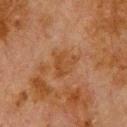workup: catalogued during a skin exam; not biopsied
size: ~3.5 mm (longest diameter)
image source: ~15 mm crop, total-body skin-cancer survey
automated metrics: about 6 CIELAB-L* units darker than the surrounding skin; a border-irregularity rating of about 5.5/10, a within-lesion color-variation index near 2/10, and radial color variation of about 0.5
tile lighting: cross-polarized illumination
subject: male, aged 78 to 82
anatomic site: the upper back